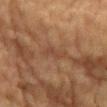Q: Was this lesion biopsied?
A: no biopsy performed (imaged during a skin exam)
Q: What is the imaging modality?
A: total-body-photography crop, ~15 mm field of view
Q: Where on the body is the lesion?
A: the front of the torso
Q: What is the lesion's diameter?
A: about 3 mm
Q: How was the tile lit?
A: cross-polarized
Q: What are the patient's age and sex?
A: male, in their mid-80s or older